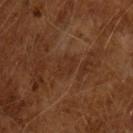| key | value |
|---|---|
| follow-up | catalogued during a skin exam; not biopsied |
| subject | male, approximately 65 years of age |
| automated lesion analysis | a nevus-likeness score of about 0/100 and a lesion-detection confidence of about 65/100 |
| acquisition | total-body-photography crop, ~15 mm field of view |
| lesion diameter | about 3 mm |